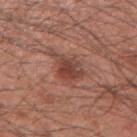follow-up: catalogued during a skin exam; not biopsied | subject: male, about 60 years old | lighting: white-light illumination | imaging modality: 15 mm crop, total-body photography | anatomic site: the right upper arm | lesion diameter: about 4.5 mm.